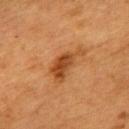Part of a total-body skin-imaging series; this lesion was reviewed on a skin check and was not flagged for biopsy.
The tile uses cross-polarized illumination.
A 15 mm close-up extracted from a 3D total-body photography capture.
Located on the back.
The total-body-photography lesion software estimated a classifier nevus-likeness of about 75/100 and a lesion-detection confidence of about 100/100.
A female patient aged approximately 55.